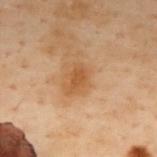biopsy status=total-body-photography surveillance lesion; no biopsy | image-analysis metrics=a lesion area of about 3 mm², an eccentricity of roughly 0.8, and a symmetry-axis asymmetry near 0.3; a mean CIELAB color near L≈46 a*≈21 b*≈36, roughly 8 lightness units darker than nearby skin, and a normalized border contrast of about 7; internal color variation of about 0.5 on a 0–10 scale and radial color variation of about 0 | location=the back | tile lighting=cross-polarized | lesion size=~2.5 mm (longest diameter) | acquisition=total-body-photography crop, ~15 mm field of view | patient=female, aged 58 to 62.